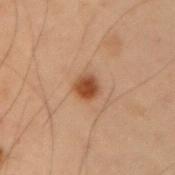{
  "biopsy_status": "not biopsied; imaged during a skin examination",
  "image": {
    "source": "total-body photography crop",
    "field_of_view_mm": 15
  },
  "patient": {
    "sex": "male",
    "age_approx": 55
  },
  "site": "right upper arm"
}